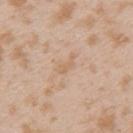Imaged during a routine full-body skin examination; the lesion was not biopsied and no histopathology is available. About 2.5 mm across. The patient is a female aged approximately 25. This is a white-light tile. A 15 mm close-up extracted from a 3D total-body photography capture. The total-body-photography lesion software estimated an area of roughly 2 mm² and a symmetry-axis asymmetry near 0.65. It also reported a classifier nevus-likeness of about 0/100 and a lesion-detection confidence of about 100/100. On the left upper arm.